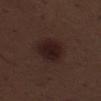| feature | finding |
|---|---|
| follow-up | total-body-photography surveillance lesion; no biopsy |
| image | ~15 mm crop, total-body skin-cancer survey |
| size | about 4.5 mm |
| automated lesion analysis | a footprint of about 10 mm², an outline eccentricity of about 0.75 (0 = round, 1 = elongated), and a symmetry-axis asymmetry near 0.15; a border-irregularity rating of about 2/10, a within-lesion color-variation index near 3/10, and a peripheral color-asymmetry measure near 1; a nevus-likeness score of about 90/100 |
| illumination | white-light |
| patient | male, roughly 70 years of age |
| location | the left thigh |